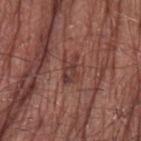workup: catalogued during a skin exam; not biopsied
patient: male, in their mid-60s
imaging modality: ~15 mm tile from a whole-body skin photo
body site: the left upper arm
lesion diameter: about 3.5 mm
lighting: white-light